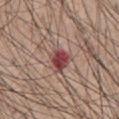• follow-up · no biopsy performed (imaged during a skin exam)
• illumination · white-light illumination
• image · ~15 mm crop, total-body skin-cancer survey
• location · the chest
• subject · male, aged 63 to 67